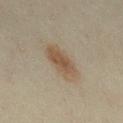Impression: Part of a total-body skin-imaging series; this lesion was reviewed on a skin check and was not flagged for biopsy. Image and clinical context: This is a cross-polarized tile. A close-up tile cropped from a whole-body skin photograph, about 15 mm across. The total-body-photography lesion software estimated roughly 9 lightness units darker than nearby skin. And it measured border irregularity of about 2.5 on a 0–10 scale, internal color variation of about 2.5 on a 0–10 scale, and peripheral color asymmetry of about 1. And it measured a classifier nevus-likeness of about 100/100 and a detector confidence of about 100 out of 100 that the crop contains a lesion. Measured at roughly 5 mm in maximum diameter. The subject is a female in their mid-30s. The lesion is located on the abdomen.